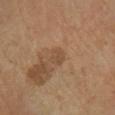{"lighting": "cross-polarized", "site": "leg", "image": {"source": "total-body photography crop", "field_of_view_mm": 15}, "lesion_size": {"long_diameter_mm_approx": 2.0}, "automated_metrics": {"cielab_L": 49, "cielab_a": 18, "cielab_b": 32, "vs_skin_darker_L": 7.0, "vs_skin_contrast_norm": 5.0, "nevus_likeness_0_100": 0, "lesion_detection_confidence_0_100": 100}, "patient": {"sex": "female", "age_approx": 70}}